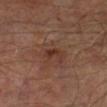Located on the right lower leg.
An algorithmic analysis of the crop reported a border-irregularity rating of about 4.5/10 and a color-variation rating of about 0.5/10. The analysis additionally found a classifier nevus-likeness of about 15/100 and a detector confidence of about 100 out of 100 that the crop contains a lesion.
Captured under cross-polarized illumination.
Longest diameter approximately 2.5 mm.
Cropped from a total-body skin-imaging series; the visible field is about 15 mm.
The subject is a male roughly 65 years of age.